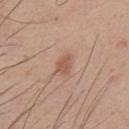biopsy status: catalogued during a skin exam; not biopsied
image-analysis metrics: a within-lesion color-variation index near 2/10 and a peripheral color-asymmetry measure near 0.5; lesion-presence confidence of about 100/100
acquisition: 15 mm crop, total-body photography
subject: male, about 25 years old
tile lighting: white-light
site: the front of the torso